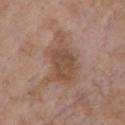• biopsy status · no biopsy performed (imaged during a skin exam)
• image · 15 mm crop, total-body photography
• patient · female, about 85 years old or older
• location · the chest
• lesion size · about 6.5 mm
• automated metrics · a footprint of about 16 mm², a shape eccentricity near 0.8, and a symmetry-axis asymmetry near 0.35; a within-lesion color-variation index near 3/10 and peripheral color asymmetry of about 1